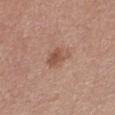- biopsy status — no biopsy performed (imaged during a skin exam)
- acquisition — 15 mm crop, total-body photography
- diameter — about 3 mm
- site — the right thigh
- subject — female, aged around 50
- lighting — white-light illumination